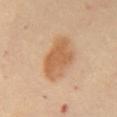Clinical impression:
The lesion was tiled from a total-body skin photograph and was not biopsied.
Background:
This image is a 15 mm lesion crop taken from a total-body photograph. The lesion-visualizer software estimated border irregularity of about 2 on a 0–10 scale, internal color variation of about 3 on a 0–10 scale, and a peripheral color-asymmetry measure near 1. Longest diameter approximately 5 mm. On the chest. The patient is a female approximately 60 years of age. Imaged with cross-polarized lighting.